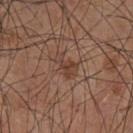<case>
  <biopsy_status>not biopsied; imaged during a skin examination</biopsy_status>
  <image>
    <source>total-body photography crop</source>
    <field_of_view_mm>15</field_of_view_mm>
  </image>
  <automated_metrics>
    <area_mm2_approx>4.5</area_mm2_approx>
    <eccentricity>0.85</eccentricity>
    <shape_asymmetry>0.4</shape_asymmetry>
    <cielab_L>41</cielab_L>
    <cielab_a>19</cielab_a>
    <cielab_b>26</cielab_b>
    <vs_skin_darker_L>7.0</vs_skin_darker_L>
    <vs_skin_contrast_norm>6.5</vs_skin_contrast_norm>
    <border_irregularity_0_10>5.0</border_irregularity_0_10>
    <peripheral_color_asymmetry>0.5</peripheral_color_asymmetry>
    <nevus_likeness_0_100>65</nevus_likeness_0_100>
  </automated_metrics>
  <lesion_size>
    <long_diameter_mm_approx>3.0</long_diameter_mm_approx>
  </lesion_size>
  <site>abdomen</site>
  <patient>
    <sex>male</sex>
    <age_approx>55</age_approx>
  </patient>
  <lighting>white-light</lighting>
</case>